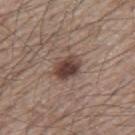This lesion was catalogued during total-body skin photography and was not selected for biopsy. Located on the mid back. Imaged with white-light lighting. A close-up tile cropped from a whole-body skin photograph, about 15 mm across. The recorded lesion diameter is about 3.5 mm. The patient is a male aged 63–67.